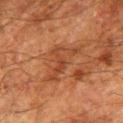| field | value |
|---|---|
| follow-up | catalogued during a skin exam; not biopsied |
| site | the leg |
| lighting | cross-polarized |
| size | about 6 mm |
| patient | male, aged 78–82 |
| image | 15 mm crop, total-body photography |